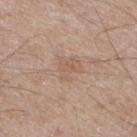notes: total-body-photography surveillance lesion; no biopsy | location: the leg | lesion diameter: ~3.5 mm (longest diameter) | image source: ~15 mm crop, total-body skin-cancer survey | subject: male, about 65 years old.